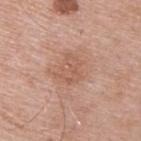biopsy status=catalogued during a skin exam; not biopsied
size=~3.5 mm (longest diameter)
site=the upper back
imaging modality=~15 mm crop, total-body skin-cancer survey
illumination=white-light illumination
subject=male, aged 63–67